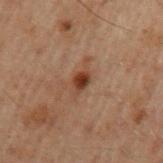Captured during whole-body skin photography for melanoma surveillance; the lesion was not biopsied.
Imaged with cross-polarized lighting.
Measured at roughly 2.5 mm in maximum diameter.
A lesion tile, about 15 mm wide, cut from a 3D total-body photograph.
A male patient, aged 58–62.
The lesion is on the left upper arm.